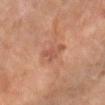Imaged during a routine full-body skin examination; the lesion was not biopsied and no histopathology is available. From the right forearm. This is a cross-polarized tile. Cropped from a whole-body photographic skin survey; the tile spans about 15 mm. A female subject, aged 48 to 52. Longest diameter approximately 3.5 mm.